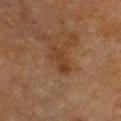workup — no biopsy performed (imaged during a skin exam); image source — total-body-photography crop, ~15 mm field of view; site — the chest; illumination — cross-polarized illumination; patient — male, aged 83 or older.